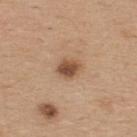Q: Was this lesion biopsied?
A: total-body-photography surveillance lesion; no biopsy
Q: What is the imaging modality?
A: ~15 mm crop, total-body skin-cancer survey
Q: What is the anatomic site?
A: the upper back
Q: Who is the patient?
A: male, in their mid-30s